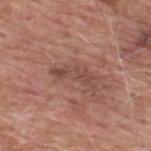<case>
  <biopsy_status>not biopsied; imaged during a skin examination</biopsy_status>
  <image>
    <source>total-body photography crop</source>
    <field_of_view_mm>15</field_of_view_mm>
  </image>
  <lesion_size>
    <long_diameter_mm_approx>5.0</long_diameter_mm_approx>
  </lesion_size>
  <site>back</site>
  <patient>
    <sex>male</sex>
    <age_approx>60</age_approx>
  </patient>
</case>